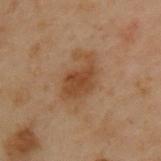No biopsy was performed on this lesion — it was imaged during a full skin examination and was not determined to be concerning.